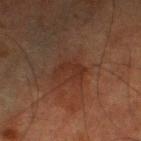{
  "biopsy_status": "not biopsied; imaged during a skin examination",
  "lesion_size": {
    "long_diameter_mm_approx": 3.5
  },
  "automated_metrics": {
    "vs_skin_contrast_norm": 5.0,
    "border_irregularity_0_10": 4.5,
    "color_variation_0_10": 1.5,
    "peripheral_color_asymmetry": 0.5
  },
  "image": {
    "source": "total-body photography crop",
    "field_of_view_mm": 15
  },
  "lighting": "cross-polarized",
  "patient": {
    "sex": "male",
    "age_approx": 75
  },
  "site": "left lower leg"
}